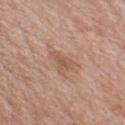Imaged during a routine full-body skin examination; the lesion was not biopsied and no histopathology is available.